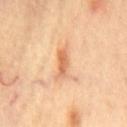- acquisition: ~15 mm crop, total-body skin-cancer survey
- tile lighting: cross-polarized
- lesion size: ≈3.5 mm
- site: the chest